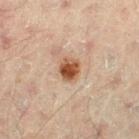Q: Was a biopsy performed?
A: imaged on a skin check; not biopsied
Q: How was the tile lit?
A: cross-polarized
Q: What kind of image is this?
A: 15 mm crop, total-body photography
Q: Where on the body is the lesion?
A: the left thigh
Q: What are the patient's age and sex?
A: male, roughly 45 years of age
Q: Lesion size?
A: about 3 mm
Q: Automated lesion metrics?
A: an average lesion color of about L≈45 a*≈20 b*≈30 (CIELAB), roughly 13 lightness units darker than nearby skin, and a normalized lesion–skin contrast near 10.5; a classifier nevus-likeness of about 95/100 and a detector confidence of about 100 out of 100 that the crop contains a lesion